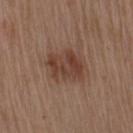Context: A 15 mm crop from a total-body photograph taken for skin-cancer surveillance. Located on the right upper arm. Measured at roughly 4.5 mm in maximum diameter. A male subject aged 68–72. This is a white-light tile.